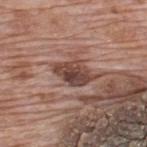| feature | finding |
|---|---|
| follow-up | total-body-photography surveillance lesion; no biopsy |
| site | the upper back |
| automated metrics | a lesion area of about 7.5 mm², a shape eccentricity near 0.85, and a symmetry-axis asymmetry near 0.25; a border-irregularity index near 2.5/10, internal color variation of about 4.5 on a 0–10 scale, and radial color variation of about 1.5; a nevus-likeness score of about 45/100 and lesion-presence confidence of about 95/100 |
| diameter | ~4 mm (longest diameter) |
| illumination | white-light illumination |
| subject | male, aged 68–72 |
| image source | total-body-photography crop, ~15 mm field of view |